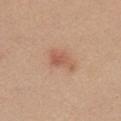notes=total-body-photography surveillance lesion; no biopsy | body site=the chest | lesion diameter=about 3.5 mm | tile lighting=white-light | image source=~15 mm crop, total-body skin-cancer survey | patient=female, roughly 45 years of age.